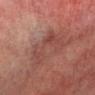Impression:
The lesion was tiled from a total-body skin photograph and was not biopsied.
Acquisition and patient details:
A male patient approximately 75 years of age. Measured at roughly 6.5 mm in maximum diameter. This image is a 15 mm lesion crop taken from a total-body photograph. An algorithmic analysis of the crop reported about 5 CIELAB-L* units darker than the surrounding skin and a normalized border contrast of about 5. It also reported a classifier nevus-likeness of about 0/100 and lesion-presence confidence of about 55/100. Captured under cross-polarized illumination. From the left lower leg.